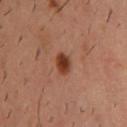Cropped from a total-body skin-imaging series; the visible field is about 15 mm. A male patient roughly 40 years of age. The lesion is on the upper back.